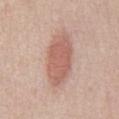Captured during whole-body skin photography for melanoma surveillance; the lesion was not biopsied.
From the abdomen.
This image is a 15 mm lesion crop taken from a total-body photograph.
Automated image analysis of the tile measured an area of roughly 17 mm², an eccentricity of roughly 0.85, and a shape-asymmetry score of about 0.1 (0 = symmetric). And it measured a border-irregularity rating of about 1.5/10 and a within-lesion color-variation index near 2/10. The software also gave an automated nevus-likeness rating near 95 out of 100.
About 6.5 mm across.
A male patient aged around 60.
The tile uses white-light illumination.